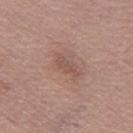Captured during whole-body skin photography for melanoma surveillance; the lesion was not biopsied. From the left thigh. A region of skin cropped from a whole-body photographic capture, roughly 15 mm wide. The subject is a male aged 53–57. Longest diameter approximately 3 mm.